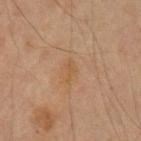Impression: The lesion was tiled from a total-body skin photograph and was not biopsied. Clinical summary: A male patient roughly 60 years of age. Longest diameter approximately 2.5 mm. Automated image analysis of the tile measured a nevus-likeness score of about 0/100 and lesion-presence confidence of about 100/100. Cropped from a whole-body photographic skin survey; the tile spans about 15 mm. From the arm.